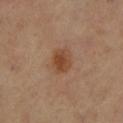Part of a total-body skin-imaging series; this lesion was reviewed on a skin check and was not flagged for biopsy. On the leg. This image is a 15 mm lesion crop taken from a total-body photograph. Automated tile analysis of the lesion measured a footprint of about 6 mm². Approximately 3 mm at its widest. The subject is a female aged 68–72.